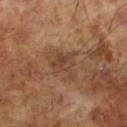Clinical impression:
This lesion was catalogued during total-body skin photography and was not selected for biopsy.
Clinical summary:
A male subject about 65 years old. Imaged with cross-polarized lighting. A 15 mm crop from a total-body photograph taken for skin-cancer surveillance. The recorded lesion diameter is about 4.5 mm.